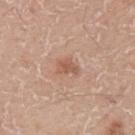Q: Who is the patient?
A: male, approximately 60 years of age
Q: What is the anatomic site?
A: the arm
Q: How was this image acquired?
A: ~15 mm tile from a whole-body skin photo